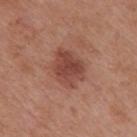<case>
  <biopsy_status>not biopsied; imaged during a skin examination</biopsy_status>
  <lesion_size>
    <long_diameter_mm_approx>4.5</long_diameter_mm_approx>
  </lesion_size>
  <site>mid back</site>
  <patient>
    <sex>male</sex>
    <age_approx>70</age_approx>
  </patient>
  <lighting>white-light</lighting>
  <automated_metrics>
    <area_mm2_approx>11.0</area_mm2_approx>
    <eccentricity>0.65</eccentricity>
    <shape_asymmetry>0.25</shape_asymmetry>
    <vs_skin_darker_L>11.0</vs_skin_darker_L>
    <vs_skin_contrast_norm>8.0</vs_skin_contrast_norm>
  </automated_metrics>
  <image>
    <source>total-body photography crop</source>
    <field_of_view_mm>15</field_of_view_mm>
  </image>
</case>